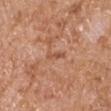notes — catalogued during a skin exam; not biopsied | lighting — white-light illumination | anatomic site — the right upper arm | TBP lesion metrics — an area of roughly 2.5 mm², a shape eccentricity near 0.9, and a symmetry-axis asymmetry near 0.35; an average lesion color of about L≈53 a*≈24 b*≈33 (CIELAB), about 8 CIELAB-L* units darker than the surrounding skin, and a lesion-to-skin contrast of about 5.5 (normalized; higher = more distinct); border irregularity of about 4.5 on a 0–10 scale, internal color variation of about 0 on a 0–10 scale, and a peripheral color-asymmetry measure near 0; a nevus-likeness score of about 0/100 and a detector confidence of about 100 out of 100 that the crop contains a lesion | diameter — ~2.5 mm (longest diameter) | patient — male, aged approximately 65 | acquisition — total-body-photography crop, ~15 mm field of view.